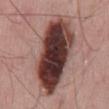A 15 mm close-up tile from a total-body photography series done for melanoma screening. The lesion's longest dimension is about 11 mm. The patient is a male in their mid- to late 60s. Automated image analysis of the tile measured roughly 24 lightness units darker than nearby skin. Captured under white-light illumination. Located on the mid back. Histopathologically confirmed as a malignant skin lesion: melanoma in situ arising in association with a nevus.